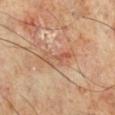Clinical impression:
Imaged during a routine full-body skin examination; the lesion was not biopsied and no histopathology is available.
Image and clinical context:
A close-up tile cropped from a whole-body skin photograph, about 15 mm across. Automated image analysis of the tile measured a lesion area of about 6 mm², a shape eccentricity near 0.95, and two-axis asymmetry of about 0.4. The lesion is on the right lower leg. Longest diameter approximately 5 mm. A male patient aged 68 to 72. Imaged with cross-polarized lighting.